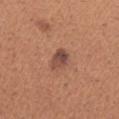- biopsy status — catalogued during a skin exam; not biopsied
- anatomic site — the right forearm
- subject — female, aged approximately 40
- image — ~15 mm tile from a whole-body skin photo